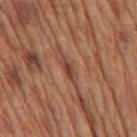Part of a total-body skin-imaging series; this lesion was reviewed on a skin check and was not flagged for biopsy.
Automated tile analysis of the lesion measured a lesion area of about 3 mm², an eccentricity of roughly 0.9, and a shape-asymmetry score of about 0.2 (0 = symmetric). And it measured a lesion–skin lightness drop of about 10 and a normalized border contrast of about 7.5.
Captured under white-light illumination.
A male patient, about 65 years old.
Approximately 3 mm at its widest.
On the mid back.
A 15 mm close-up extracted from a 3D total-body photography capture.